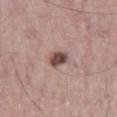biopsy_status: not biopsied; imaged during a skin examination
image:
  source: total-body photography crop
  field_of_view_mm: 15
site: right thigh
patient:
  sex: male
  age_approx: 40
lighting: white-light